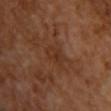follow-up = catalogued during a skin exam; not biopsied
image = total-body-photography crop, ~15 mm field of view
illumination = cross-polarized illumination
patient = female
anatomic site = the chest